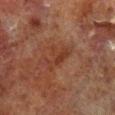acquisition: 15 mm crop, total-body photography | subject: male, roughly 70 years of age | tile lighting: cross-polarized | lesion size: ~4 mm (longest diameter) | location: the right lower leg.